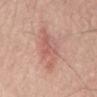A roughly 15 mm field-of-view crop from a total-body skin photograph.
Automated tile analysis of the lesion measured border irregularity of about 4 on a 0–10 scale and a peripheral color-asymmetry measure near 1.5.
A male patient aged approximately 70.
The lesion is located on the mid back.
The tile uses white-light illumination.